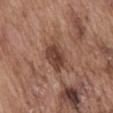Q: Was a biopsy performed?
A: no biopsy performed (imaged during a skin exam)
Q: What kind of image is this?
A: ~15 mm crop, total-body skin-cancer survey
Q: What is the anatomic site?
A: the abdomen
Q: Patient demographics?
A: male, aged around 75